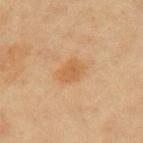Imaged with cross-polarized lighting.
About 3.5 mm across.
A lesion tile, about 15 mm wide, cut from a 3D total-body photograph.
On the left upper arm.
A female subject aged 48–52.
The total-body-photography lesion software estimated an area of roughly 6.5 mm². The software also gave a lesion–skin lightness drop of about 6 and a lesion-to-skin contrast of about 6 (normalized; higher = more distinct). It also reported border irregularity of about 2 on a 0–10 scale, a within-lesion color-variation index near 2/10, and a peripheral color-asymmetry measure near 0.5. It also reported a classifier nevus-likeness of about 50/100 and a detector confidence of about 100 out of 100 that the crop contains a lesion.